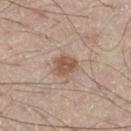Impression: The lesion was photographed on a routine skin check and not biopsied; there is no pathology result. Context: The subject is a male about 60 years old. A region of skin cropped from a whole-body photographic capture, roughly 15 mm wide. The lesion's longest dimension is about 3 mm. On the left thigh. This is a white-light tile. The lesion-visualizer software estimated an average lesion color of about L≈54 a*≈18 b*≈27 (CIELAB), a lesion–skin lightness drop of about 11, and a lesion-to-skin contrast of about 7.5 (normalized; higher = more distinct).